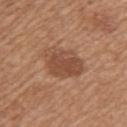Clinical impression: Part of a total-body skin-imaging series; this lesion was reviewed on a skin check and was not flagged for biopsy. Background: Longest diameter approximately 5 mm. The tile uses white-light illumination. The lesion is on the arm. A roughly 15 mm field-of-view crop from a total-body skin photograph. Automated tile analysis of the lesion measured border irregularity of about 2.5 on a 0–10 scale, a color-variation rating of about 3/10, and peripheral color asymmetry of about 1. The software also gave a detector confidence of about 100 out of 100 that the crop contains a lesion. The patient is a female aged approximately 55.